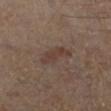Q: Was a biopsy performed?
A: total-body-photography surveillance lesion; no biopsy
Q: Automated lesion metrics?
A: a classifier nevus-likeness of about 0/100 and a detector confidence of about 100 out of 100 that the crop contains a lesion
Q: Illumination type?
A: cross-polarized
Q: Patient demographics?
A: approximately 55 years of age
Q: How was this image acquired?
A: ~15 mm tile from a whole-body skin photo
Q: How large is the lesion?
A: about 4 mm
Q: Lesion location?
A: the left lower leg